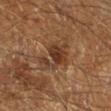Impression:
No biopsy was performed on this lesion — it was imaged during a full skin examination and was not determined to be concerning.
Acquisition and patient details:
A male subject approximately 60 years of age. Cropped from a total-body skin-imaging series; the visible field is about 15 mm. Longest diameter approximately 4.5 mm. Captured under cross-polarized illumination. The lesion is located on the leg.